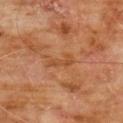Q: Was a biopsy performed?
A: catalogued during a skin exam; not biopsied
Q: Automated lesion metrics?
A: an area of roughly 3.5 mm², a shape eccentricity near 0.9, and two-axis asymmetry of about 0.3; a lesion color around L≈47 a*≈24 b*≈38 in CIELAB and a normalized border contrast of about 5.5; a border-irregularity rating of about 4.5/10 and a color-variation rating of about 0/10; an automated nevus-likeness rating near 0 out of 100
Q: How was this image acquired?
A: 15 mm crop, total-body photography
Q: Lesion location?
A: the chest
Q: Illumination type?
A: cross-polarized illumination
Q: What are the patient's age and sex?
A: male, in their 60s